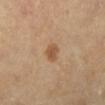* workup · catalogued during a skin exam; not biopsied
* tile lighting · cross-polarized illumination
* site · the left lower leg
* imaging modality · ~15 mm crop, total-body skin-cancer survey
* size · ≈2.5 mm
* patient · female, approximately 60 years of age